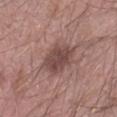Q: Was this lesion biopsied?
A: total-body-photography surveillance lesion; no biopsy
Q: How was the tile lit?
A: white-light illumination
Q: Automated lesion metrics?
A: a footprint of about 11 mm² and a shape eccentricity near 0.75; roughly 11 lightness units darker than nearby skin and a normalized border contrast of about 8.5; a nevus-likeness score of about 45/100 and a detector confidence of about 100 out of 100 that the crop contains a lesion
Q: What is the anatomic site?
A: the left forearm
Q: What kind of image is this?
A: ~15 mm crop, total-body skin-cancer survey
Q: Patient demographics?
A: male, aged 18 to 22